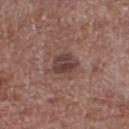This lesion was catalogued during total-body skin photography and was not selected for biopsy. Cropped from a total-body skin-imaging series; the visible field is about 15 mm. A male patient approximately 70 years of age. Automated image analysis of the tile measured a border-irregularity rating of about 2/10, internal color variation of about 4 on a 0–10 scale, and a peripheral color-asymmetry measure near 1.5. And it measured an automated nevus-likeness rating near 30 out of 100 and a detector confidence of about 100 out of 100 that the crop contains a lesion. Located on the right lower leg.